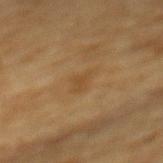This lesion was catalogued during total-body skin photography and was not selected for biopsy. The lesion is located on the mid back. The lesion's longest dimension is about 3 mm. Cropped from a total-body skin-imaging series; the visible field is about 15 mm. The subject is a male approximately 85 years of age or older. The lesion-visualizer software estimated a mean CIELAB color near L≈40 a*≈15 b*≈33, a lesion–skin lightness drop of about 5, and a normalized lesion–skin contrast near 4.5. This is a cross-polarized tile.